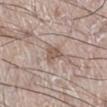Assessment:
The lesion was tiled from a total-body skin photograph and was not biopsied.
Clinical summary:
Automated tile analysis of the lesion measured a footprint of about 4 mm² and a symmetry-axis asymmetry near 0.4. It also reported a lesion–skin lightness drop of about 10 and a normalized lesion–skin contrast near 7.5. It also reported lesion-presence confidence of about 100/100. A male subject, in their 70s. On the left leg. The lesion's longest dimension is about 2.5 mm. A lesion tile, about 15 mm wide, cut from a 3D total-body photograph.